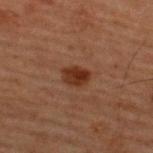biopsy status: imaged on a skin check; not biopsied | image source: total-body-photography crop, ~15 mm field of view | size: about 3 mm | tile lighting: cross-polarized | image-analysis metrics: an outline eccentricity of about 0.7 (0 = round, 1 = elongated) and a shape-asymmetry score of about 0.2 (0 = symmetric); a lesion color around L≈24 a*≈19 b*≈25 in CIELAB and a lesion-to-skin contrast of about 10 (normalized; higher = more distinct); a border-irregularity index near 1.5/10, a within-lesion color-variation index near 3.5/10, and a peripheral color-asymmetry measure near 1.5; an automated nevus-likeness rating near 90 out of 100 and a detector confidence of about 100 out of 100 that the crop contains a lesion | anatomic site: the upper back | patient: male, aged 58–62.